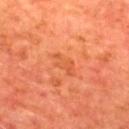Impression:
Imaged during a routine full-body skin examination; the lesion was not biopsied and no histopathology is available.
Context:
Captured under cross-polarized illumination. The lesion is located on the mid back. A close-up tile cropped from a whole-body skin photograph, about 15 mm across. The subject is a male in their mid- to late 60s. About 3.5 mm across.